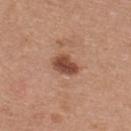| field | value |
|---|---|
| workup | imaged on a skin check; not biopsied |
| tile lighting | white-light |
| location | the upper back |
| patient | female, in their mid-30s |
| image source | total-body-photography crop, ~15 mm field of view |
| lesion size | ~3.5 mm (longest diameter) |